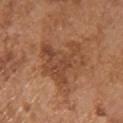This lesion was catalogued during total-body skin photography and was not selected for biopsy.
The lesion-visualizer software estimated a lesion area of about 19 mm², an eccentricity of roughly 0.7, and a shape-asymmetry score of about 0.55 (0 = symmetric). The software also gave a mean CIELAB color near L≈46 a*≈23 b*≈33, about 8 CIELAB-L* units darker than the surrounding skin, and a lesion-to-skin contrast of about 6.5 (normalized; higher = more distinct). The software also gave a color-variation rating of about 4.5/10. It also reported a nevus-likeness score of about 5/100.
On the chest.
This image is a 15 mm lesion crop taken from a total-body photograph.
A male patient in their 70s.
Approximately 6 mm at its widest.